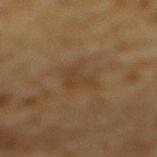<case>
<biopsy_status>not biopsied; imaged during a skin examination</biopsy_status>
<site>mid back</site>
<image>
  <source>total-body photography crop</source>
  <field_of_view_mm>15</field_of_view_mm>
</image>
<automated_metrics>
  <eccentricity>0.55</eccentricity>
  <shape_asymmetry>0.3</shape_asymmetry>
  <border_irregularity_0_10>4.0</border_irregularity_0_10>
  <color_variation_0_10>2.0</color_variation_0_10>
  <peripheral_color_asymmetry>0.5</peripheral_color_asymmetry>
</automated_metrics>
<lesion_size>
  <long_diameter_mm_approx>3.5</long_diameter_mm_approx>
</lesion_size>
<patient>
  <sex>male</sex>
  <age_approx>85+</age_approx>
</patient>
<lighting>cross-polarized</lighting>
</case>